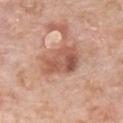Captured during whole-body skin photography for melanoma surveillance; the lesion was not biopsied. Captured under white-light illumination. A male patient aged around 60. A region of skin cropped from a whole-body photographic capture, roughly 15 mm wide. From the chest. The total-body-photography lesion software estimated a lesion area of about 13 mm² and two-axis asymmetry of about 0.3. And it measured a border-irregularity index near 3.5/10 and a within-lesion color-variation index near 6/10. The recorded lesion diameter is about 5 mm.